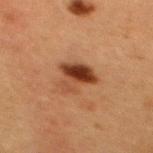Clinical summary:
From the mid back. A lesion tile, about 15 mm wide, cut from a 3D total-body photograph. Approximately 4 mm at its widest. A female patient, approximately 40 years of age. Captured under cross-polarized illumination.